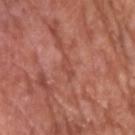Imaged during a routine full-body skin examination; the lesion was not biopsied and no histopathology is available.
The lesion is on the right upper arm.
The patient is a male roughly 75 years of age.
A 15 mm close-up extracted from a 3D total-body photography capture.
The tile uses white-light illumination.
The total-body-photography lesion software estimated an average lesion color of about L≈49 a*≈28 b*≈29 (CIELAB).